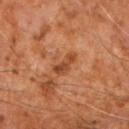Q: Is there a histopathology result?
A: catalogued during a skin exam; not biopsied
Q: Where on the body is the lesion?
A: the right forearm
Q: What did automated image analysis measure?
A: an average lesion color of about L≈43 a*≈25 b*≈35 (CIELAB), roughly 9 lightness units darker than nearby skin, and a lesion-to-skin contrast of about 7.5 (normalized; higher = more distinct); a border-irregularity index near 4/10, a within-lesion color-variation index near 1.5/10, and a peripheral color-asymmetry measure near 0.5
Q: How large is the lesion?
A: about 3.5 mm
Q: Patient demographics?
A: male, aged 58 to 62
Q: How was the tile lit?
A: cross-polarized
Q: How was this image acquired?
A: 15 mm crop, total-body photography